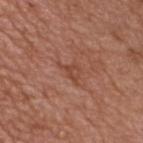Captured during whole-body skin photography for melanoma surveillance; the lesion was not biopsied.
From the chest.
A male subject approximately 50 years of age.
About 3.5 mm across.
This is a white-light tile.
A 15 mm close-up tile from a total-body photography series done for melanoma screening.